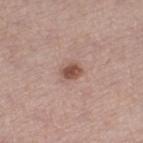notes: imaged on a skin check; not biopsied | patient: male, aged 53 to 57 | image-analysis metrics: a lesion color around L≈52 a*≈20 b*≈26 in CIELAB, roughly 12 lightness units darker than nearby skin, and a normalized border contrast of about 8.5; a color-variation rating of about 2.5/10 and radial color variation of about 1; a lesion-detection confidence of about 100/100 | lesion diameter: ≈2.5 mm | illumination: white-light | acquisition: ~15 mm crop, total-body skin-cancer survey | anatomic site: the leg.